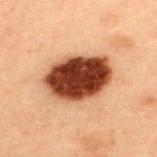Recorded during total-body skin imaging; not selected for excision or biopsy. The subject is a male aged 53–57. The total-body-photography lesion software estimated a footprint of about 26 mm². The software also gave a border-irregularity index near 1.5/10 and a peripheral color-asymmetry measure near 2.5. It also reported a nevus-likeness score of about 100/100. A 15 mm close-up extracted from a 3D total-body photography capture. The lesion is located on the back. Captured under cross-polarized illumination. Approximately 7.5 mm at its widest.